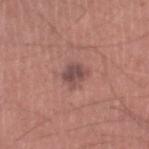The subject is a male aged around 45. The lesion is on the left lower leg. A 15 mm close-up tile from a total-body photography series done for melanoma screening.